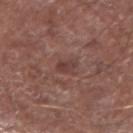{"biopsy_status": "not biopsied; imaged during a skin examination", "patient": {"sex": "male", "age_approx": 65}, "image": {"source": "total-body photography crop", "field_of_view_mm": 15}, "lighting": "white-light", "lesion_size": {"long_diameter_mm_approx": 2.5}, "site": "left forearm"}